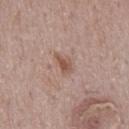Imaged during a routine full-body skin examination; the lesion was not biopsied and no histopathology is available. The recorded lesion diameter is about 3 mm. The lesion is located on the mid back. Captured under white-light illumination. A 15 mm close-up extracted from a 3D total-body photography capture. The subject is a male aged 68–72. The lesion-visualizer software estimated a lesion color around L≈53 a*≈19 b*≈26 in CIELAB and a lesion-to-skin contrast of about 7 (normalized; higher = more distinct). It also reported a color-variation rating of about 1.5/10 and radial color variation of about 0.5.